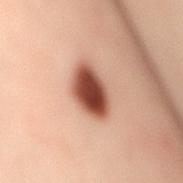Impression: This lesion was catalogued during total-body skin photography and was not selected for biopsy. Context: On the mid back. The patient is a female approximately 40 years of age. Cropped from a total-body skin-imaging series; the visible field is about 15 mm. Captured under cross-polarized illumination.